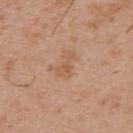workup: total-body-photography surveillance lesion; no biopsy
lighting: white-light illumination
imaging modality: total-body-photography crop, ~15 mm field of view
image-analysis metrics: a footprint of about 4 mm² and a shape-asymmetry score of about 0.55 (0 = symmetric); a nevus-likeness score of about 0/100 and a lesion-detection confidence of about 100/100
body site: the upper back
patient: male, aged 28–32